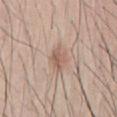Impression: The lesion was photographed on a routine skin check and not biopsied; there is no pathology result. Background: A male subject in their mid- to late 20s. The recorded lesion diameter is about 3 mm. Automated image analysis of the tile measured a classifier nevus-likeness of about 70/100 and lesion-presence confidence of about 100/100. This is a white-light tile. A region of skin cropped from a whole-body photographic capture, roughly 15 mm wide. On the abdomen.